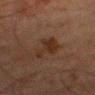| key | value |
|---|---|
| image | 15 mm crop, total-body photography |
| patient | male, roughly 80 years of age |
| location | the right thigh |